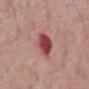{
  "biopsy_status": "not biopsied; imaged during a skin examination",
  "lighting": "white-light",
  "image": {
    "source": "total-body photography crop",
    "field_of_view_mm": 15
  },
  "automated_metrics": {
    "border_irregularity_0_10": 1.5,
    "color_variation_0_10": 4.0,
    "peripheral_color_asymmetry": 1.5
  },
  "site": "mid back",
  "lesion_size": {
    "long_diameter_mm_approx": 3.5
  },
  "patient": {
    "sex": "male",
    "age_approx": 65
  }
}